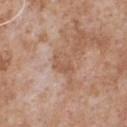biopsy_status: not biopsied; imaged during a skin examination
lighting: white-light
patient:
  sex: male
  age_approx: 65
lesion_size:
  long_diameter_mm_approx: 3.0
site: chest
image:
  source: total-body photography crop
  field_of_view_mm: 15
automated_metrics:
  cielab_L: 56
  cielab_a: 20
  cielab_b: 31
  vs_skin_darker_L: 7.0
  vs_skin_contrast_norm: 5.5
  nevus_likeness_0_100: 0
  lesion_detection_confidence_0_100: 100